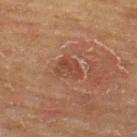Imaged during a routine full-body skin examination; the lesion was not biopsied and no histopathology is available. On the upper back. A lesion tile, about 15 mm wide, cut from a 3D total-body photograph. Approximately 3 mm at its widest. Captured under cross-polarized illumination. A male patient, aged 63–67.